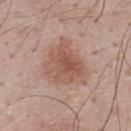Part of a total-body skin-imaging series; this lesion was reviewed on a skin check and was not flagged for biopsy. Located on the upper back. Cropped from a whole-body photographic skin survey; the tile spans about 15 mm. The patient is a male aged around 45.